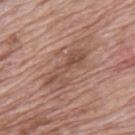This is a white-light tile.
Automated image analysis of the tile measured a nevus-likeness score of about 0/100 and a lesion-detection confidence of about 100/100.
The lesion is located on the mid back.
A male patient approximately 70 years of age.
A 15 mm crop from a total-body photograph taken for skin-cancer surveillance.